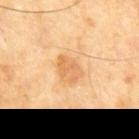{
  "biopsy_status": "not biopsied; imaged during a skin examination",
  "image": {
    "source": "total-body photography crop",
    "field_of_view_mm": 15
  },
  "lighting": "cross-polarized",
  "automated_metrics": {
    "cielab_L": 66,
    "cielab_a": 21,
    "cielab_b": 42,
    "vs_skin_darker_L": 8.0,
    "border_irregularity_0_10": 1.5,
    "color_variation_0_10": 3.5,
    "nevus_likeness_0_100": 0
  },
  "site": "abdomen",
  "patient": {
    "sex": "male",
    "age_approx": 65
  }
}